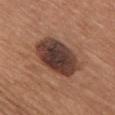Findings:
- follow-up · imaged on a skin check; not biopsied
- body site · the chest
- size · ≈7 mm
- subject · female, aged approximately 65
- imaging modality · total-body-photography crop, ~15 mm field of view